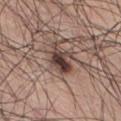Notes:
• workup — total-body-photography surveillance lesion; no biopsy
• imaging modality — 15 mm crop, total-body photography
• automated metrics — a lesion color around L≈40 a*≈17 b*≈21 in CIELAB and roughly 15 lightness units darker than nearby skin; a border-irregularity rating of about 5/10, a color-variation rating of about 5.5/10, and peripheral color asymmetry of about 1.5; a nevus-likeness score of about 90/100 and a detector confidence of about 95 out of 100 that the crop contains a lesion
• diameter — ≈4.5 mm
• anatomic site — the lower back
• patient — male, in their 70s
• lighting — white-light